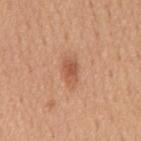Impression: No biopsy was performed on this lesion — it was imaged during a full skin examination and was not determined to be concerning. Acquisition and patient details: A male patient, aged 53–57. The tile uses white-light illumination. The lesion is located on the mid back. A 15 mm crop from a total-body photograph taken for skin-cancer surveillance. Longest diameter approximately 2.5 mm.